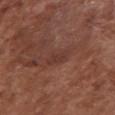Clinical impression:
Part of a total-body skin-imaging series; this lesion was reviewed on a skin check and was not flagged for biopsy.
Context:
Approximately 3 mm at its widest. A lesion tile, about 15 mm wide, cut from a 3D total-body photograph. A female patient roughly 75 years of age. Captured under white-light illumination. The lesion is located on the chest.